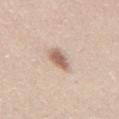| field | value |
|---|---|
| follow-up | no biopsy performed (imaged during a skin exam) |
| diameter | ≈3 mm |
| patient | male, about 45 years old |
| acquisition | total-body-photography crop, ~15 mm field of view |
| location | the abdomen |